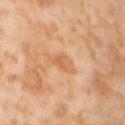follow-up = total-body-photography surveillance lesion; no biopsy
patient = female, about 55 years old
image source = ~15 mm tile from a whole-body skin photo
location = the left thigh